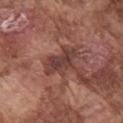workup: no biopsy performed (imaged during a skin exam)
imaging modality: ~15 mm tile from a whole-body skin photo
subject: male, in their mid-70s
location: the right upper arm
lesion size: ≈4.5 mm
illumination: white-light